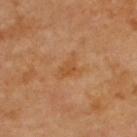Q: Is there a histopathology result?
A: no biopsy performed (imaged during a skin exam)
Q: What kind of image is this?
A: 15 mm crop, total-body photography
Q: Who is the patient?
A: male, in their 70s
Q: Lesion location?
A: the upper back